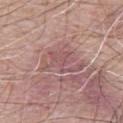notes: imaged on a skin check; not biopsied | image-analysis metrics: an average lesion color of about L≈54 a*≈22 b*≈20 (CIELAB) and roughly 8 lightness units darker than nearby skin; a border-irregularity rating of about 7.5/10, internal color variation of about 4 on a 0–10 scale, and peripheral color asymmetry of about 1; a nevus-likeness score of about 0/100 and a lesion-detection confidence of about 90/100 | tile lighting: white-light | anatomic site: the abdomen | patient: male, roughly 60 years of age | image source: total-body-photography crop, ~15 mm field of view | lesion size: about 6 mm.